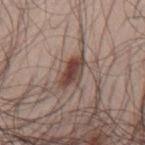Assessment:
Imaged during a routine full-body skin examination; the lesion was not biopsied and no histopathology is available.
Context:
A region of skin cropped from a whole-body photographic capture, roughly 15 mm wide. A male patient aged around 50. Located on the mid back.